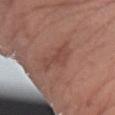Assessment: Part of a total-body skin-imaging series; this lesion was reviewed on a skin check and was not flagged for biopsy. Context: The tile uses white-light illumination. The lesion's longest dimension is about 4.5 mm. A 15 mm crop from a total-body photograph taken for skin-cancer surveillance. An algorithmic analysis of the crop reported a lesion area of about 6.5 mm², an outline eccentricity of about 0.9 (0 = round, 1 = elongated), and two-axis asymmetry of about 0.45. It also reported an average lesion color of about L≈46 a*≈23 b*≈25 (CIELAB), roughly 7 lightness units darker than nearby skin, and a lesion-to-skin contrast of about 5 (normalized; higher = more distinct). It also reported a classifier nevus-likeness of about 5/100. The lesion is on the left forearm. A female patient aged around 75.